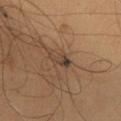Impression:
This lesion was catalogued during total-body skin photography and was not selected for biopsy.
Background:
An algorithmic analysis of the crop reported a shape eccentricity near 0.85. The patient is a male in their mid-60s. On the chest. Imaged with cross-polarized lighting. A region of skin cropped from a whole-body photographic capture, roughly 15 mm wide. About 3 mm across.